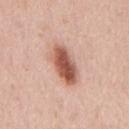biopsy_status: not biopsied; imaged during a skin examination
image:
  source: total-body photography crop
  field_of_view_mm: 15
site: chest
patient:
  sex: male
  age_approx: 65
lesion_size:
  long_diameter_mm_approx: 5.5